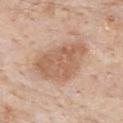biopsy status: imaged on a skin check; not biopsied
patient: male, aged 83 or older
image: total-body-photography crop, ~15 mm field of view
TBP lesion metrics: a lesion-detection confidence of about 100/100
lesion diameter: about 7 mm
illumination: white-light illumination
body site: the upper back